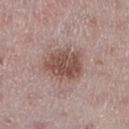{
  "biopsy_status": "not biopsied; imaged during a skin examination",
  "patient": {
    "sex": "female",
    "age_approx": 45
  },
  "lesion_size": {
    "long_diameter_mm_approx": 5.0
  },
  "site": "left lower leg",
  "image": {
    "source": "total-body photography crop",
    "field_of_view_mm": 15
  },
  "automated_metrics": {
    "cielab_L": 50,
    "cielab_a": 19,
    "cielab_b": 23,
    "vs_skin_darker_L": 12.0,
    "vs_skin_contrast_norm": 8.5
  }
}